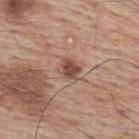Assessment: No biopsy was performed on this lesion — it was imaged during a full skin examination and was not determined to be concerning. Image and clinical context: The total-body-photography lesion software estimated an area of roughly 5 mm², a shape eccentricity near 0.45, and a symmetry-axis asymmetry near 0.2. A male subject approximately 70 years of age. The lesion's longest dimension is about 2.5 mm. A region of skin cropped from a whole-body photographic capture, roughly 15 mm wide. Located on the upper back.